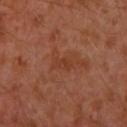Recorded during total-body skin imaging; not selected for excision or biopsy. The subject is a male approximately 30 years of age. This is a cross-polarized tile. Located on the left upper arm. About 2.5 mm across. A lesion tile, about 15 mm wide, cut from a 3D total-body photograph.